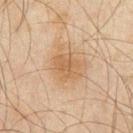{"biopsy_status": "not biopsied; imaged during a skin examination", "site": "abdomen", "patient": {"sex": "male", "age_approx": 45}, "image": {"source": "total-body photography crop", "field_of_view_mm": 15}}